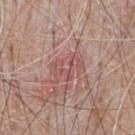Assessment:
Recorded during total-body skin imaging; not selected for excision or biopsy.
Acquisition and patient details:
The subject is a male in their mid-60s. The lesion is on the chest. The lesion-visualizer software estimated a lesion-detection confidence of about 80/100. Cropped from a whole-body photographic skin survey; the tile spans about 15 mm. Captured under white-light illumination.